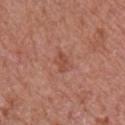Recorded during total-body skin imaging; not selected for excision or biopsy.